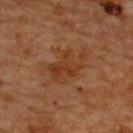From the upper back.
A male subject, in their mid-60s.
A region of skin cropped from a whole-body photographic capture, roughly 15 mm wide.